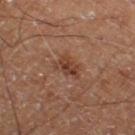Assessment: Part of a total-body skin-imaging series; this lesion was reviewed on a skin check and was not flagged for biopsy. Context: A 15 mm crop from a total-body photograph taken for skin-cancer surveillance. Approximately 3.5 mm at its widest. The patient is a male roughly 60 years of age. This is a cross-polarized tile. The lesion-visualizer software estimated an average lesion color of about L≈35 a*≈19 b*≈26 (CIELAB) and a lesion-to-skin contrast of about 7.5 (normalized; higher = more distinct). The lesion is on the right thigh.